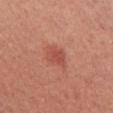| feature | finding |
|---|---|
| subject | female, aged 33 to 37 |
| acquisition | total-body-photography crop, ~15 mm field of view |
| site | the upper back |